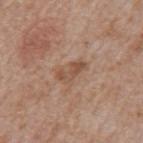Clinical impression:
Part of a total-body skin-imaging series; this lesion was reviewed on a skin check and was not flagged for biopsy.
Background:
The lesion is located on the mid back. Longest diameter approximately 3.5 mm. Automated image analysis of the tile measured a mean CIELAB color near L≈51 a*≈20 b*≈29, roughly 9 lightness units darker than nearby skin, and a normalized border contrast of about 6.5. The analysis additionally found an automated nevus-likeness rating near 0 out of 100. This is a white-light tile. A region of skin cropped from a whole-body photographic capture, roughly 15 mm wide. The patient is a male approximately 65 years of age.